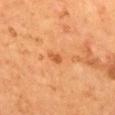Impression:
The lesion was photographed on a routine skin check and not biopsied; there is no pathology result.
Acquisition and patient details:
A 15 mm crop from a total-body photograph taken for skin-cancer surveillance. The subject is a male aged approximately 55. Located on the back.